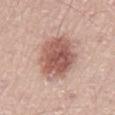{
  "biopsy_status": "not biopsied; imaged during a skin examination",
  "patient": {
    "sex": "male",
    "age_approx": 50
  },
  "site": "lower back",
  "lesion_size": {
    "long_diameter_mm_approx": 6.5
  },
  "image": {
    "source": "total-body photography crop",
    "field_of_view_mm": 15
  },
  "automated_metrics": {
    "area_mm2_approx": 22.0,
    "eccentricity": 0.7,
    "border_irregularity_0_10": 1.5,
    "color_variation_0_10": 5.5,
    "peripheral_color_asymmetry": 2.0,
    "nevus_likeness_0_100": 90,
    "lesion_detection_confidence_0_100": 100
  },
  "lighting": "white-light"
}